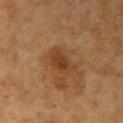No biopsy was performed on this lesion — it was imaged during a full skin examination and was not determined to be concerning.
The recorded lesion diameter is about 3.5 mm.
Cropped from a whole-body photographic skin survey; the tile spans about 15 mm.
A female patient, aged 58 to 62.
On the right upper arm.